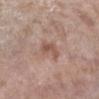<tbp_lesion>
  <biopsy_status>not biopsied; imaged during a skin examination</biopsy_status>
  <site>left lower leg</site>
  <lesion_size>
    <long_diameter_mm_approx>2.5</long_diameter_mm_approx>
  </lesion_size>
  <image>
    <source>total-body photography crop</source>
    <field_of_view_mm>15</field_of_view_mm>
  </image>
  <patient>
    <sex>female</sex>
    <age_approx>75</age_approx>
  </patient>
  <automated_metrics>
    <area_mm2_approx>4.5</area_mm2_approx>
    <eccentricity>0.7</eccentricity>
    <shape_asymmetry>0.45</shape_asymmetry>
    <nevus_likeness_0_100>0</nevus_likeness_0_100>
    <lesion_detection_confidence_0_100>100</lesion_detection_confidence_0_100>
  </automated_metrics>
</tbp_lesion>